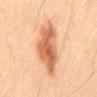notes: total-body-photography surveillance lesion; no biopsy | acquisition: ~15 mm crop, total-body skin-cancer survey | subject: male, aged approximately 35 | location: the mid back | tile lighting: cross-polarized illumination | automated metrics: a classifier nevus-likeness of about 95/100 and lesion-presence confidence of about 100/100 | size: ~7.5 mm (longest diameter).